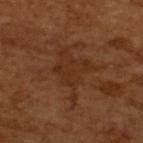{
  "biopsy_status": "not biopsied; imaged during a skin examination",
  "lighting": "cross-polarized",
  "lesion_size": {
    "long_diameter_mm_approx": 6.5
  },
  "image": {
    "source": "total-body photography crop",
    "field_of_view_mm": 15
  },
  "automated_metrics": {
    "peripheral_color_asymmetry": 0.5,
    "nevus_likeness_0_100": 0,
    "lesion_detection_confidence_0_100": 85
  },
  "patient": {
    "sex": "male",
    "age_approx": 65
  }
}